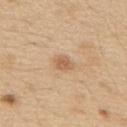<lesion>
  <image>
    <source>total-body photography crop</source>
    <field_of_view_mm>15</field_of_view_mm>
  </image>
  <automated_metrics>
    <border_irregularity_0_10>1.5</border_irregularity_0_10>
    <color_variation_0_10>2.5</color_variation_0_10>
    <peripheral_color_asymmetry>1.0</peripheral_color_asymmetry>
  </automated_metrics>
  <site>upper back</site>
  <patient>
    <sex>male</sex>
    <age_approx>70</age_approx>
  </patient>
  <lesion_size>
    <long_diameter_mm_approx>2.5</long_diameter_mm_approx>
  </lesion_size>
</lesion>